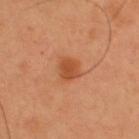No biopsy was performed on this lesion — it was imaged during a full skin examination and was not determined to be concerning.
Automated image analysis of the tile measured a footprint of about 4.5 mm², an outline eccentricity of about 0.55 (0 = round, 1 = elongated), and a symmetry-axis asymmetry near 0.2. The software also gave an average lesion color of about L≈48 a*≈27 b*≈38 (CIELAB) and a lesion-to-skin contrast of about 7.5 (normalized; higher = more distinct). And it measured a border-irregularity rating of about 2/10, a color-variation rating of about 1.5/10, and a peripheral color-asymmetry measure near 0.5. It also reported a classifier nevus-likeness of about 80/100.
From the upper back.
About 2.5 mm across.
Imaged with cross-polarized lighting.
Cropped from a whole-body photographic skin survey; the tile spans about 15 mm.
A male subject aged around 55.